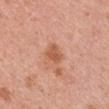Q: Is there a histopathology result?
A: no biopsy performed (imaged during a skin exam)
Q: Where on the body is the lesion?
A: the left upper arm
Q: What are the patient's age and sex?
A: female, roughly 50 years of age
Q: How was this image acquired?
A: total-body-photography crop, ~15 mm field of view